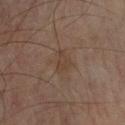Part of a total-body skin-imaging series; this lesion was reviewed on a skin check and was not flagged for biopsy.
The lesion is on the left forearm.
Captured under cross-polarized illumination.
A region of skin cropped from a whole-body photographic capture, roughly 15 mm wide.
The patient is a male about 70 years old.
The lesion-visualizer software estimated an eccentricity of roughly 0.75 and a symmetry-axis asymmetry near 0.45. And it measured border irregularity of about 4.5 on a 0–10 scale, a within-lesion color-variation index near 2/10, and a peripheral color-asymmetry measure near 1. It also reported a classifier nevus-likeness of about 0/100.